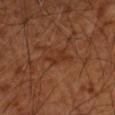Part of a total-body skin-imaging series; this lesion was reviewed on a skin check and was not flagged for biopsy. Approximately 3 mm at its widest. The lesion is located on the left forearm. Imaged with cross-polarized lighting. A subject about 65 years old. This image is a 15 mm lesion crop taken from a total-body photograph.